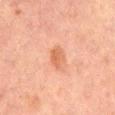biopsy status: no biopsy performed (imaged during a skin exam)
subject: male, aged around 65
image source: 15 mm crop, total-body photography
site: the abdomen